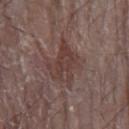Captured during whole-body skin photography for melanoma surveillance; the lesion was not biopsied.
Cropped from a total-body skin-imaging series; the visible field is about 15 mm.
A male subject aged around 80.
Measured at roughly 5 mm in maximum diameter.
The tile uses white-light illumination.
From the arm.
An algorithmic analysis of the crop reported a footprint of about 12 mm², an eccentricity of roughly 0.75, and a shape-asymmetry score of about 0.35 (0 = symmetric). The software also gave lesion-presence confidence of about 100/100.